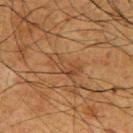  biopsy_status: not biopsied; imaged during a skin examination
  image:
    source: total-body photography crop
    field_of_view_mm: 15
  site: upper back
  patient:
    sex: male
    age_approx: 60
  lesion_size:
    long_diameter_mm_approx: 4.0